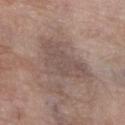notes = catalogued during a skin exam; not biopsied | subject = female, aged approximately 70 | illumination = white-light | image source = total-body-photography crop, ~15 mm field of view | size = ~5.5 mm (longest diameter) | location = the left lower leg.